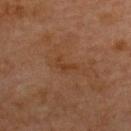A 15 mm close-up tile from a total-body photography series done for melanoma screening.
The lesion's longest dimension is about 2.5 mm.
A female subject, aged 68–72.
Located on the upper back.